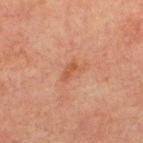Impression: The lesion was tiled from a total-body skin photograph and was not biopsied. Acquisition and patient details: The total-body-photography lesion software estimated a lesion area of about 3.5 mm² and two-axis asymmetry of about 0.4. It also reported border irregularity of about 4.5 on a 0–10 scale, a within-lesion color-variation index near 1/10, and peripheral color asymmetry of about 0. The lesion is located on the upper back. A 15 mm crop from a total-body photograph taken for skin-cancer surveillance. A male patient, in their 60s.